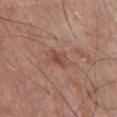Part of a total-body skin-imaging series; this lesion was reviewed on a skin check and was not flagged for biopsy.
Captured under white-light illumination.
This image is a 15 mm lesion crop taken from a total-body photograph.
A male patient aged 68 to 72.
Measured at roughly 2.5 mm in maximum diameter.
On the chest.